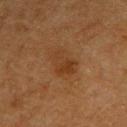Captured during whole-body skin photography for melanoma surveillance; the lesion was not biopsied. The recorded lesion diameter is about 4 mm. The tile uses cross-polarized illumination. A male patient in their mid-70s. A region of skin cropped from a whole-body photographic capture, roughly 15 mm wide. The total-body-photography lesion software estimated a lesion color around L≈30 a*≈18 b*≈29 in CIELAB, roughly 6 lightness units darker than nearby skin, and a normalized border contrast of about 6. And it measured a border-irregularity index near 3.5/10, a within-lesion color-variation index near 3.5/10, and radial color variation of about 1.5. The software also gave a lesion-detection confidence of about 100/100. The lesion is on the back.